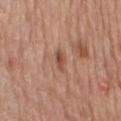Clinical impression:
Recorded during total-body skin imaging; not selected for excision or biopsy.
Background:
This is a white-light tile. A male subject aged 68–72. Cropped from a whole-body photographic skin survey; the tile spans about 15 mm. The lesion is on the left thigh.